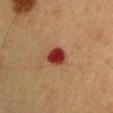Case summary:
- notes — imaged on a skin check; not biopsied
- lesion diameter — ≈2.5 mm
- image source — ~15 mm tile from a whole-body skin photo
- anatomic site — the left upper arm
- subject — male, aged approximately 55
- lighting — cross-polarized illumination